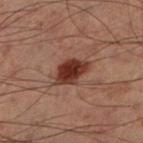<record>
  <biopsy_status>not biopsied; imaged during a skin examination</biopsy_status>
  <patient>
    <sex>male</sex>
    <age_approx>60</age_approx>
  </patient>
  <lighting>cross-polarized</lighting>
  <image>
    <source>total-body photography crop</source>
    <field_of_view_mm>15</field_of_view_mm>
  </image>
  <lesion_size>
    <long_diameter_mm_approx>4.0</long_diameter_mm_approx>
  </lesion_size>
  <site>left lower leg</site>
</record>